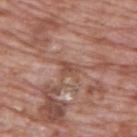Recorded during total-body skin imaging; not selected for excision or biopsy.
A male subject, about 70 years old.
A close-up tile cropped from a whole-body skin photograph, about 15 mm across.
About 2.5 mm across.
The lesion is on the upper back.
This is a white-light tile.
Automated tile analysis of the lesion measured a mean CIELAB color near L≈49 a*≈22 b*≈28, about 8 CIELAB-L* units darker than the surrounding skin, and a normalized border contrast of about 6.5. The software also gave a border-irregularity index near 4.5/10 and a peripheral color-asymmetry measure near 0.5. It also reported a classifier nevus-likeness of about 0/100 and lesion-presence confidence of about 85/100.